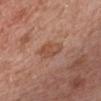This lesion was catalogued during total-body skin photography and was not selected for biopsy.